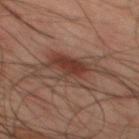Clinical impression: The lesion was photographed on a routine skin check and not biopsied; there is no pathology result. Background: A male patient aged 43–47. A 15 mm close-up tile from a total-body photography series done for melanoma screening. On the mid back.